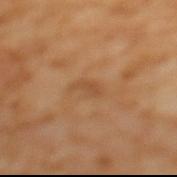workup — no biopsy performed (imaged during a skin exam) | image source — 15 mm crop, total-body photography | illumination — cross-polarized illumination | patient — female, aged around 55 | automated metrics — a border-irregularity rating of about 4/10; lesion-presence confidence of about 100/100 | anatomic site — the mid back.